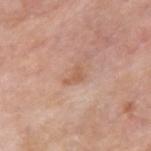Q: Was a biopsy performed?
A: no biopsy performed (imaged during a skin exam)